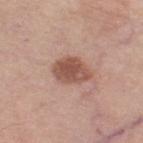The lesion was photographed on a routine skin check and not biopsied; there is no pathology result.
Automated image analysis of the tile measured a footprint of about 9.5 mm², a shape eccentricity near 0.7, and two-axis asymmetry of about 0.15. And it measured a lesion–skin lightness drop of about 13 and a normalized lesion–skin contrast near 8.5. And it measured a color-variation rating of about 3/10.
Captured under white-light illumination.
Cropped from a total-body skin-imaging series; the visible field is about 15 mm.
From the right thigh.
Longest diameter approximately 4 mm.
A female subject aged approximately 65.